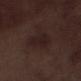Assessment: Captured during whole-body skin photography for melanoma surveillance; the lesion was not biopsied. Context: The patient is a male aged 68 to 72. On the left lower leg. Cropped from a total-body skin-imaging series; the visible field is about 15 mm.